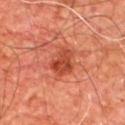| key | value |
|---|---|
| follow-up | total-body-photography surveillance lesion; no biopsy |
| automated metrics | a footprint of about 7 mm², a shape eccentricity near 0.75, and a shape-asymmetry score of about 0.35 (0 = symmetric); a border-irregularity index near 3.5/10, internal color variation of about 4.5 on a 0–10 scale, and peripheral color asymmetry of about 1.5; a lesion-detection confidence of about 100/100 |
| patient | male, approximately 65 years of age |
| image | 15 mm crop, total-body photography |
| site | the front of the torso |